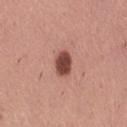The lesion was tiled from a total-body skin photograph and was not biopsied. A female subject about 40 years old. Located on the right thigh. A lesion tile, about 15 mm wide, cut from a 3D total-body photograph. The lesion's longest dimension is about 3 mm.